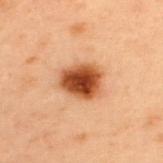The lesion was tiled from a total-body skin photograph and was not biopsied. Captured under cross-polarized illumination. A lesion tile, about 15 mm wide, cut from a 3D total-body photograph. The subject is a male approximately 55 years of age. Located on the upper back. Longest diameter approximately 4 mm.